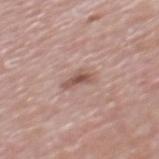Recorded during total-body skin imaging; not selected for excision or biopsy.
This is a white-light tile.
Automated tile analysis of the lesion measured a shape-asymmetry score of about 0.5 (0 = symmetric). The analysis additionally found a mean CIELAB color near L≈54 a*≈20 b*≈24, about 11 CIELAB-L* units darker than the surrounding skin, and a normalized border contrast of about 7.5. And it measured internal color variation of about 2.5 on a 0–10 scale and a peripheral color-asymmetry measure near 0.5. The software also gave an automated nevus-likeness rating near 30 out of 100 and a detector confidence of about 100 out of 100 that the crop contains a lesion.
A close-up tile cropped from a whole-body skin photograph, about 15 mm across.
The subject is a male about 70 years old.
The lesion is located on the mid back.
Longest diameter approximately 3 mm.